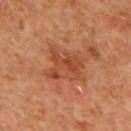Findings:
• workup: catalogued during a skin exam; not biopsied
• lesion size: about 5.5 mm
• imaging modality: ~15 mm crop, total-body skin-cancer survey
• tile lighting: cross-polarized
• subject: male, aged around 65
• site: the back